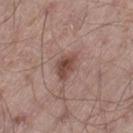Case summary:
– workup: total-body-photography surveillance lesion; no biopsy
– image: ~15 mm tile from a whole-body skin photo
– size: ≈3 mm
– site: the left thigh
– subject: male, aged approximately 55
– tile lighting: white-light